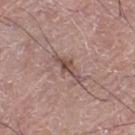On the leg. A region of skin cropped from a whole-body photographic capture, roughly 15 mm wide. The recorded lesion diameter is about 4.5 mm. A male subject approximately 75 years of age. Captured under white-light illumination.